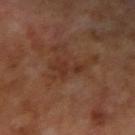workup: total-body-photography surveillance lesion; no biopsy
anatomic site: the right upper arm
automated lesion analysis: an area of roughly 5 mm², an outline eccentricity of about 0.9 (0 = round, 1 = elongated), and a symmetry-axis asymmetry near 0.5
lesion size: ≈4.5 mm
imaging modality: ~15 mm tile from a whole-body skin photo
illumination: cross-polarized
patient: male, aged 68–72